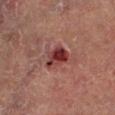biopsy_status: not biopsied; imaged during a skin examination
automated_metrics:
  area_mm2_approx: 6.0
  shape_asymmetry: 0.25
  color_variation_0_10: 6.0
  peripheral_color_asymmetry: 2.0
patient:
  sex: male
  age_approx: 55
image:
  source: total-body photography crop
  field_of_view_mm: 15
site: left lower leg
lesion_size:
  long_diameter_mm_approx: 3.5
lighting: cross-polarized